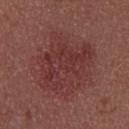{"biopsy_status": "not biopsied; imaged during a skin examination", "image": {"source": "total-body photography crop", "field_of_view_mm": 15}, "patient": {"sex": "female", "age_approx": 25}, "site": "upper back"}